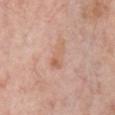Findings:
- notes: imaged on a skin check; not biopsied
- image source: ~15 mm crop, total-body skin-cancer survey
- size: ~3.5 mm (longest diameter)
- anatomic site: the chest
- automated metrics: about 7 CIELAB-L* units darker than the surrounding skin and a lesion-to-skin contrast of about 5.5 (normalized; higher = more distinct); a border-irregularity rating of about 4/10, a color-variation rating of about 2.5/10, and peripheral color asymmetry of about 0.5; an automated nevus-likeness rating near 0 out of 100 and a lesion-detection confidence of about 100/100
- patient: male, aged around 60